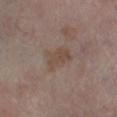patient:
  sex: male
  age_approx: 70
site: left lower leg
image:
  source: total-body photography crop
  field_of_view_mm: 15
automated_metrics:
  area_mm2_approx: 7.0
  eccentricity: 0.8
  shape_asymmetry: 0.3
  cielab_L: 44
  cielab_a: 14
  cielab_b: 23
  vs_skin_darker_L: 6.0
  vs_skin_contrast_norm: 5.5
  nevus_likeness_0_100: 0
lighting: cross-polarized
lesion_size:
  long_diameter_mm_approx: 4.0